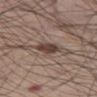biopsy status = no biopsy performed (imaged during a skin exam)
body site = the left thigh
tile lighting = white-light illumination
automated metrics = a lesion area of about 6 mm² and a symmetry-axis asymmetry near 0.15; internal color variation of about 3.5 on a 0–10 scale
imaging modality = total-body-photography crop, ~15 mm field of view
lesion size = ≈3 mm
patient = male, in their mid-50s